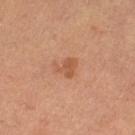imaging modality: ~15 mm tile from a whole-body skin photo; diameter: ≈2.5 mm; subject: female, in their 60s; TBP lesion metrics: lesion-presence confidence of about 100/100; location: the left thigh.